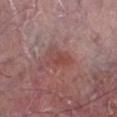{"biopsy_status": "not biopsied; imaged during a skin examination", "lighting": "white-light", "site": "left lower leg", "automated_metrics": {"area_mm2_approx": 6.5, "eccentricity": 0.75, "border_irregularity_0_10": 3.5, "color_variation_0_10": 4.0, "peripheral_color_asymmetry": 1.5, "nevus_likeness_0_100": 20}, "patient": {"sex": "male", "age_approx": 40}, "lesion_size": {"long_diameter_mm_approx": 3.5}, "image": {"source": "total-body photography crop", "field_of_view_mm": 15}}